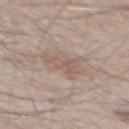Imaged during a routine full-body skin examination; the lesion was not biopsied and no histopathology is available. A male subject approximately 55 years of age. Located on the mid back. This image is a 15 mm lesion crop taken from a total-body photograph.